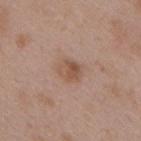Clinical impression:
Imaged during a routine full-body skin examination; the lesion was not biopsied and no histopathology is available.
Image and clinical context:
This image is a 15 mm lesion crop taken from a total-body photograph. Automated image analysis of the tile measured a lesion–skin lightness drop of about 8 and a normalized border contrast of about 6. The analysis additionally found a border-irregularity rating of about 1.5/10. It also reported a classifier nevus-likeness of about 30/100 and a lesion-detection confidence of about 100/100. This is a white-light tile. The lesion is located on the mid back. The subject is a male aged around 50.